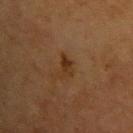The lesion was photographed on a routine skin check and not biopsied; there is no pathology result. Approximately 3 mm at its widest. A female subject, aged 38–42. A close-up tile cropped from a whole-body skin photograph, about 15 mm across. The lesion is located on the back.